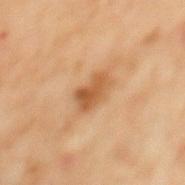biopsy status = total-body-photography surveillance lesion; no biopsy | lesion diameter = ~3.5 mm (longest diameter) | illumination = cross-polarized illumination | image source = total-body-photography crop, ~15 mm field of view | anatomic site = the mid back | subject = male, aged 58 to 62 | automated metrics = a symmetry-axis asymmetry near 0.2; border irregularity of about 2.5 on a 0–10 scale, a within-lesion color-variation index near 3.5/10, and a peripheral color-asymmetry measure near 1.5.